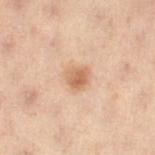Notes:
– notes — imaged on a skin check; not biopsied